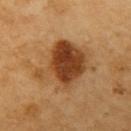• workup · imaged on a skin check; not biopsied
• acquisition · total-body-photography crop, ~15 mm field of view
• location · the left upper arm
• patient · male, aged 58 to 62
• lesion diameter · ~7 mm (longest diameter)
• tile lighting · cross-polarized illumination
• automated lesion analysis · an average lesion color of about L≈43 a*≈22 b*≈37 (CIELAB) and a lesion–skin lightness drop of about 14